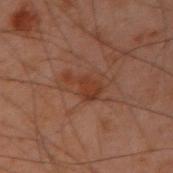The lesion was photographed on a routine skin check and not biopsied; there is no pathology result.
Measured at roughly 4 mm in maximum diameter.
Cropped from a whole-body photographic skin survey; the tile spans about 15 mm.
A male patient, aged 48–52.
This is a cross-polarized tile.
An algorithmic analysis of the crop reported a lesion area of about 5 mm². The analysis additionally found border irregularity of about 6 on a 0–10 scale, internal color variation of about 2 on a 0–10 scale, and radial color variation of about 0.5. It also reported an automated nevus-likeness rating near 5 out of 100 and a detector confidence of about 100 out of 100 that the crop contains a lesion.
The lesion is located on the left arm.